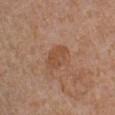Case summary:
– workup · no biopsy performed (imaged during a skin exam)
– anatomic site · the left lower leg
– lesion size · ~4 mm (longest diameter)
– subject · female, aged approximately 55
– image · ~15 mm tile from a whole-body skin photo
– image-analysis metrics · a mean CIELAB color near L≈49 a*≈21 b*≈33, about 7 CIELAB-L* units darker than the surrounding skin, and a lesion-to-skin contrast of about 6 (normalized; higher = more distinct); border irregularity of about 2 on a 0–10 scale and a within-lesion color-variation index near 2.5/10; a nevus-likeness score of about 0/100 and lesion-presence confidence of about 100/100